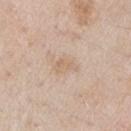Q: Was this lesion biopsied?
A: total-body-photography surveillance lesion; no biopsy
Q: What did automated image analysis measure?
A: a footprint of about 4 mm² and an outline eccentricity of about 0.8 (0 = round, 1 = elongated); an average lesion color of about L≈65 a*≈15 b*≈30 (CIELAB), roughly 6 lightness units darker than nearby skin, and a normalized border contrast of about 5; a border-irregularity index near 3/10 and internal color variation of about 1.5 on a 0–10 scale; a nevus-likeness score of about 0/100 and a lesion-detection confidence of about 100/100
Q: What is the anatomic site?
A: the arm
Q: What is the lesion's diameter?
A: ~3 mm (longest diameter)
Q: Who is the patient?
A: male, approximately 55 years of age
Q: What is the imaging modality?
A: total-body-photography crop, ~15 mm field of view